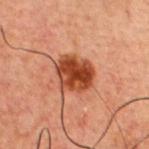• notes — total-body-photography surveillance lesion; no biopsy
• tile lighting — cross-polarized
• subject — male, about 60 years old
• body site — the chest
• automated lesion analysis — an average lesion color of about L≈35 a*≈24 b*≈30 (CIELAB), a lesion–skin lightness drop of about 13, and a normalized border contrast of about 11.5
• acquisition — 15 mm crop, total-body photography